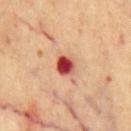Captured during whole-body skin photography for melanoma surveillance; the lesion was not biopsied. The patient is a male roughly 70 years of age. The tile uses cross-polarized illumination. An algorithmic analysis of the crop reported about 20 CIELAB-L* units darker than the surrounding skin and a lesion-to-skin contrast of about 13.5 (normalized; higher = more distinct). And it measured a border-irregularity rating of about 1/10, a color-variation rating of about 8/10, and peripheral color asymmetry of about 2. And it measured a nevus-likeness score of about 0/100 and a detector confidence of about 100 out of 100 that the crop contains a lesion. The recorded lesion diameter is about 2.5 mm. A close-up tile cropped from a whole-body skin photograph, about 15 mm across. The lesion is located on the chest.